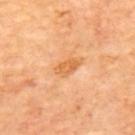Image and clinical context:
Automated image analysis of the tile measured an area of roughly 4.5 mm², an eccentricity of roughly 0.85, and a shape-asymmetry score of about 0.2 (0 = symmetric). A close-up tile cropped from a whole-body skin photograph, about 15 mm across. A subject aged 63–67. From the upper back. Imaged with cross-polarized lighting.